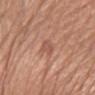Q: Is there a histopathology result?
A: no biopsy performed (imaged during a skin exam)
Q: How large is the lesion?
A: about 2.5 mm
Q: What did automated image analysis measure?
A: border irregularity of about 4 on a 0–10 scale, internal color variation of about 1 on a 0–10 scale, and peripheral color asymmetry of about 0.5; an automated nevus-likeness rating near 0 out of 100 and a detector confidence of about 100 out of 100 that the crop contains a lesion
Q: What is the imaging modality?
A: ~15 mm crop, total-body skin-cancer survey
Q: Illumination type?
A: white-light
Q: What is the anatomic site?
A: the left forearm
Q: What are the patient's age and sex?
A: female, aged around 65